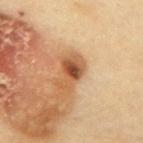notes = imaged on a skin check; not biopsied | imaging modality = ~15 mm crop, total-body skin-cancer survey | subject = female, aged 58 to 62 | lesion diameter = ≈4 mm | illumination = cross-polarized illumination | anatomic site = the upper back.